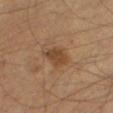notes = imaged on a skin check; not biopsied
lighting = cross-polarized illumination
subject = male, aged around 60
size = ≈3 mm
acquisition = ~15 mm tile from a whole-body skin photo
anatomic site = the leg